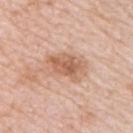No biopsy was performed on this lesion — it was imaged during a full skin examination and was not determined to be concerning. A female patient in their mid-40s. Located on the upper back. Imaged with white-light lighting. A 15 mm close-up extracted from a 3D total-body photography capture. The recorded lesion diameter is about 5 mm.